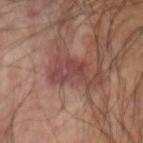  biopsy_status: not biopsied; imaged during a skin examination
  lighting: cross-polarized
  image:
    source: total-body photography crop
    field_of_view_mm: 15
  patient:
    age_approx: 55
  site: left forearm